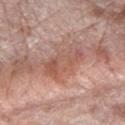Clinical impression: No biopsy was performed on this lesion — it was imaged during a full skin examination and was not determined to be concerning. Clinical summary: A female patient roughly 70 years of age. An algorithmic analysis of the crop reported a lesion area of about 12 mm² and two-axis asymmetry of about 0.25. A close-up tile cropped from a whole-body skin photograph, about 15 mm across. The tile uses white-light illumination. Located on the right forearm. Longest diameter approximately 6 mm.